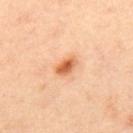| feature | finding |
|---|---|
| notes | catalogued during a skin exam; not biopsied |
| patient | male, aged 43–47 |
| image source | total-body-photography crop, ~15 mm field of view |
| body site | the mid back |